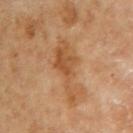No biopsy was performed on this lesion — it was imaged during a full skin examination and was not determined to be concerning. A female subject, in their 60s. The lesion is on the right upper arm. Imaged with cross-polarized lighting. Cropped from a total-body skin-imaging series; the visible field is about 15 mm. The lesion's longest dimension is about 6.5 mm.